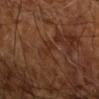Recorded during total-body skin imaging; not selected for excision or biopsy. The total-body-photography lesion software estimated an area of roughly 1.5 mm², an eccentricity of roughly 0.95, and a shape-asymmetry score of about 0.5 (0 = symmetric). It also reported a lesion–skin lightness drop of about 5 and a normalized border contrast of about 5.5. And it measured an automated nevus-likeness rating near 0 out of 100 and a lesion-detection confidence of about 95/100. The lesion is located on the right upper arm. A region of skin cropped from a whole-body photographic capture, roughly 15 mm wide. Measured at roughly 2.5 mm in maximum diameter. The subject is aged approximately 65. Imaged with cross-polarized lighting.